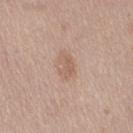{
  "biopsy_status": "not biopsied; imaged during a skin examination",
  "image": {
    "source": "total-body photography crop",
    "field_of_view_mm": 15
  },
  "site": "right thigh",
  "lesion_size": {
    "long_diameter_mm_approx": 3.5
  },
  "automated_metrics": {
    "area_mm2_approx": 4.5,
    "shape_asymmetry": 0.35,
    "border_irregularity_0_10": 4.5,
    "color_variation_0_10": 2.0,
    "peripheral_color_asymmetry": 0.5
  },
  "lighting": "white-light",
  "patient": {
    "sex": "female",
    "age_approx": 40
  }
}